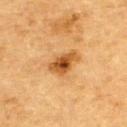Findings:
– workup: total-body-photography surveillance lesion; no biopsy
– site: the upper back
– TBP lesion metrics: an average lesion color of about L≈48 a*≈22 b*≈41 (CIELAB), about 13 CIELAB-L* units darker than the surrounding skin, and a normalized border contrast of about 9.5; a border-irregularity rating of about 3.5/10 and internal color variation of about 6 on a 0–10 scale; a nevus-likeness score of about 90/100 and a detector confidence of about 100 out of 100 that the crop contains a lesion
– image: ~15 mm crop, total-body skin-cancer survey
– lesion diameter: ~4 mm (longest diameter)
– subject: male, about 85 years old or older